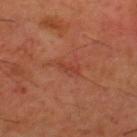The lesion was photographed on a routine skin check and not biopsied; there is no pathology result. From the upper back. The lesion's longest dimension is about 3 mm. The subject is a male roughly 60 years of age. Imaged with cross-polarized lighting. A lesion tile, about 15 mm wide, cut from a 3D total-body photograph.